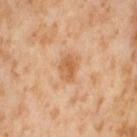Assessment:
The lesion was photographed on a routine skin check and not biopsied; there is no pathology result.